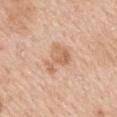biopsy_status: not biopsied; imaged during a skin examination
lesion_size:
  long_diameter_mm_approx: 3.5
patient:
  sex: male
  age_approx: 60
site: mid back
image:
  source: total-body photography crop
  field_of_view_mm: 15
lighting: white-light